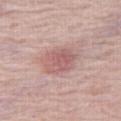{
  "biopsy_status": "not biopsied; imaged during a skin examination",
  "lighting": "white-light",
  "site": "right thigh",
  "lesion_size": {
    "long_diameter_mm_approx": 4.0
  },
  "image": {
    "source": "total-body photography crop",
    "field_of_view_mm": 15
  },
  "patient": {
    "sex": "female",
    "age_approx": 60
  }
}